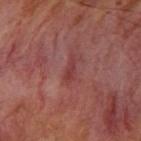<lesion>
  <biopsy_status>not biopsied; imaged during a skin examination</biopsy_status>
  <lighting>cross-polarized</lighting>
  <lesion_size>
    <long_diameter_mm_approx>3.5</long_diameter_mm_approx>
  </lesion_size>
  <image>
    <source>total-body photography crop</source>
    <field_of_view_mm>15</field_of_view_mm>
  </image>
  <site>right upper arm</site>
  <patient>
    <sex>male</sex>
    <age_approx>55</age_approx>
  </patient>
</lesion>